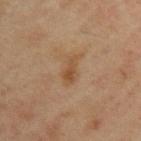Impression:
Part of a total-body skin-imaging series; this lesion was reviewed on a skin check and was not flagged for biopsy.
Clinical summary:
The subject is a male aged around 45. The lesion is on the arm. A lesion tile, about 15 mm wide, cut from a 3D total-body photograph. Captured under cross-polarized illumination. Approximately 3.5 mm at its widest.